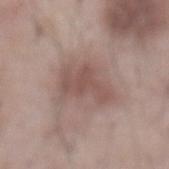Part of a total-body skin-imaging series; this lesion was reviewed on a skin check and was not flagged for biopsy.
On the abdomen.
Cropped from a total-body skin-imaging series; the visible field is about 15 mm.
A male subject, in their mid-50s.